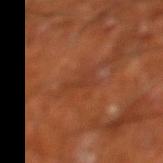Recorded during total-body skin imaging; not selected for excision or biopsy. The tile uses cross-polarized illumination. A male subject approximately 65 years of age. The lesion is on the leg. Longest diameter approximately 3 mm. Automated image analysis of the tile measured a lesion-detection confidence of about 60/100. Cropped from a whole-body photographic skin survey; the tile spans about 15 mm.